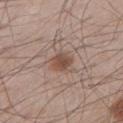biopsy status: no biopsy performed (imaged during a skin exam); lighting: white-light illumination; acquisition: total-body-photography crop, ~15 mm field of view; location: the left lower leg; patient: male, about 60 years old; diameter: ~2.5 mm (longest diameter).